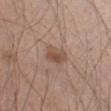Findings:
• workup — no biopsy performed (imaged during a skin exam)
• subject — male, aged around 45
• automated lesion analysis — a lesion color around L≈50 a*≈18 b*≈27 in CIELAB, roughly 9 lightness units darker than nearby skin, and a normalized lesion–skin contrast near 7; a border-irregularity index near 2/10 and peripheral color asymmetry of about 1; a nevus-likeness score of about 85/100 and a lesion-detection confidence of about 100/100
• image — 15 mm crop, total-body photography
• lesion diameter — about 3 mm
• lighting — white-light illumination
• anatomic site — the right forearm